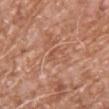notes = catalogued during a skin exam; not biopsied | image = ~15 mm tile from a whole-body skin photo | subject = male, aged 58–62 | location = the upper back | lesion size = ~2.5 mm (longest diameter) | image-analysis metrics = a mean CIELAB color near L≈54 a*≈24 b*≈33, about 6 CIELAB-L* units darker than the surrounding skin, and a normalized lesion–skin contrast near 5.